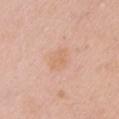workup = no biopsy performed (imaged during a skin exam); diameter = ~3.5 mm (longest diameter); anatomic site = the chest; tile lighting = white-light illumination; subject = female, aged around 65; imaging modality = total-body-photography crop, ~15 mm field of view.